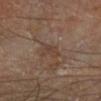Recorded during total-body skin imaging; not selected for excision or biopsy. The patient is a male aged 68–72. Located on the leg. A 15 mm close-up tile from a total-body photography series done for melanoma screening. The total-body-photography lesion software estimated an area of roughly 4 mm², an eccentricity of roughly 0.85, and two-axis asymmetry of about 0.3. The software also gave a lesion color around L≈39 a*≈16 b*≈24 in CIELAB and a normalized border contrast of about 5. The analysis additionally found a within-lesion color-variation index near 2/10.